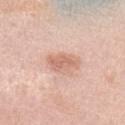Clinical impression: The lesion was photographed on a routine skin check and not biopsied; there is no pathology result. Image and clinical context: Imaged with white-light lighting. A male patient aged 28 to 32. A 15 mm close-up tile from a total-body photography series done for melanoma screening.